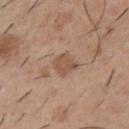follow-up — imaged on a skin check; not biopsied
patient — male, roughly 40 years of age
anatomic site — the chest
automated lesion analysis — a footprint of about 5.5 mm² and a shape eccentricity near 0.45; border irregularity of about 2.5 on a 0–10 scale, a color-variation rating of about 2/10, and radial color variation of about 1
diameter — ≈2.5 mm
image — ~15 mm crop, total-body skin-cancer survey
tile lighting — white-light illumination